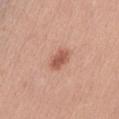Assessment: The lesion was tiled from a total-body skin photograph and was not biopsied.